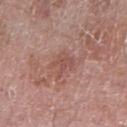{"biopsy_status": "not biopsied; imaged during a skin examination", "image": {"source": "total-body photography crop", "field_of_view_mm": 15}, "patient": {"sex": "male", "age_approx": 60}, "site": "right forearm"}